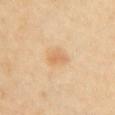Recorded during total-body skin imaging; not selected for excision or biopsy. The subject is a female aged approximately 40. A close-up tile cropped from a whole-body skin photograph, about 15 mm across. The lesion is located on the chest. The tile uses cross-polarized illumination. Measured at roughly 2.5 mm in maximum diameter.